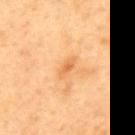Findings:
- notes · catalogued during a skin exam; not biopsied
- size · ~3 mm (longest diameter)
- image · ~15 mm tile from a whole-body skin photo
- location · the back
- lighting · cross-polarized illumination
- subject · male, aged around 50